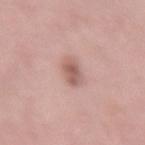Recorded during total-body skin imaging; not selected for excision or biopsy. The subject is a male about 55 years old. From the lower back. A region of skin cropped from a whole-body photographic capture, roughly 15 mm wide.